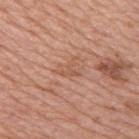tile lighting: white-light; size: ~3 mm (longest diameter); body site: the right upper arm; subject: female, aged approximately 45; acquisition: 15 mm crop, total-body photography.